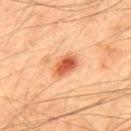Clinical impression: No biopsy was performed on this lesion — it was imaged during a full skin examination and was not determined to be concerning. Background: On the upper back. Measured at roughly 3.5 mm in maximum diameter. A 15 mm close-up extracted from a 3D total-body photography capture. A male subject, about 65 years old. The tile uses cross-polarized illumination.